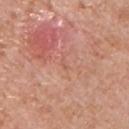subject — male, aged around 70
body site — the chest
tile lighting — white-light illumination
automated lesion analysis — a footprint of about 1 mm², a shape eccentricity near 0.9, and a symmetry-axis asymmetry near 0.5; a lesion color around L≈58 a*≈26 b*≈30 in CIELAB, a lesion–skin lightness drop of about 5, and a lesion-to-skin contrast of about 3.5 (normalized; higher = more distinct); a border-irregularity rating of about 4/10, a color-variation rating of about 0/10, and peripheral color asymmetry of about 0; an automated nevus-likeness rating near 0 out of 100 and lesion-presence confidence of about 100/100
acquisition — ~15 mm crop, total-body skin-cancer survey
lesion diameter — about 1.5 mm
diagnosis — a nodular basal cell carcinoma